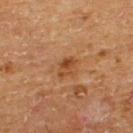notes=total-body-photography surveillance lesion; no biopsy | imaging modality=15 mm crop, total-body photography | patient=male, about 65 years old | body site=the upper back.